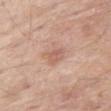Notes:
* subject · male, roughly 65 years of age
* image · ~15 mm tile from a whole-body skin photo
* location · the right thigh
* illumination · white-light
* lesion size · about 2.5 mm